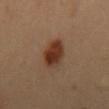Q: Where on the body is the lesion?
A: the lower back
Q: What kind of image is this?
A: 15 mm crop, total-body photography
Q: Automated lesion metrics?
A: a footprint of about 9.5 mm², a shape eccentricity near 0.8, and a symmetry-axis asymmetry near 0.1; an average lesion color of about L≈35 a*≈20 b*≈29 (CIELAB) and about 12 CIELAB-L* units darker than the surrounding skin; border irregularity of about 1.5 on a 0–10 scale, a within-lesion color-variation index near 5.5/10, and radial color variation of about 1.5; an automated nevus-likeness rating near 100 out of 100 and a detector confidence of about 100 out of 100 that the crop contains a lesion
Q: Patient demographics?
A: male, about 40 years old